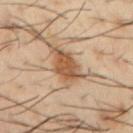Assessment: Part of a total-body skin-imaging series; this lesion was reviewed on a skin check and was not flagged for biopsy. Acquisition and patient details: About 5.5 mm across. A male patient, in their 40s. Captured under cross-polarized illumination. A 15 mm close-up tile from a total-body photography series done for melanoma screening. The lesion is on the right upper arm.